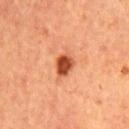{
  "biopsy_status": "not biopsied; imaged during a skin examination",
  "patient": {
    "sex": "male",
    "age_approx": 65
  },
  "automated_metrics": {
    "border_irregularity_0_10": 2.0,
    "color_variation_0_10": 3.0,
    "peripheral_color_asymmetry": 1.0
  },
  "image": {
    "source": "total-body photography crop",
    "field_of_view_mm": 15
  },
  "site": "chest",
  "lighting": "cross-polarized"
}